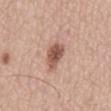Captured during whole-body skin photography for melanoma surveillance; the lesion was not biopsied. A male subject, aged around 65. On the mid back. Cropped from a whole-body photographic skin survey; the tile spans about 15 mm. Imaged with white-light lighting. Measured at roughly 4 mm in maximum diameter. The lesion-visualizer software estimated a footprint of about 6.5 mm², an eccentricity of roughly 0.85, and a symmetry-axis asymmetry near 0.3. It also reported a lesion color around L≈54 a*≈22 b*≈27 in CIELAB, roughly 14 lightness units darker than nearby skin, and a normalized border contrast of about 9.5. It also reported border irregularity of about 3 on a 0–10 scale, internal color variation of about 4 on a 0–10 scale, and peripheral color asymmetry of about 1.5.